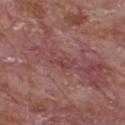Case summary:
- notes · imaged on a skin check; not biopsied
- diameter · about 2.5 mm
- acquisition · total-body-photography crop, ~15 mm field of view
- patient · male, about 65 years old
- body site · the front of the torso